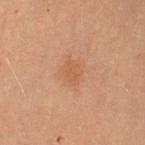A lesion tile, about 15 mm wide, cut from a 3D total-body photograph. The recorded lesion diameter is about 3 mm. The lesion is located on the arm. A female subject, aged 68 to 72. This is a cross-polarized tile.